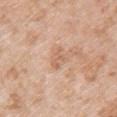Automated image analysis of the tile measured an automated nevus-likeness rating near 0 out of 100. Imaged with white-light lighting. A female subject about 70 years old. On the left upper arm. A 15 mm close-up tile from a total-body photography series done for melanoma screening. Approximately 3 mm at its widest.